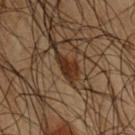Part of a total-body skin-imaging series; this lesion was reviewed on a skin check and was not flagged for biopsy. Approximately 2.5 mm at its widest. The total-body-photography lesion software estimated an outline eccentricity of about 0.8 (0 = round, 1 = elongated). The software also gave an average lesion color of about L≈21 a*≈15 b*≈23 (CIELAB), roughly 9 lightness units darker than nearby skin, and a lesion-to-skin contrast of about 10.5 (normalized; higher = more distinct). And it measured an automated nevus-likeness rating near 85 out of 100 and a detector confidence of about 95 out of 100 that the crop contains a lesion. A male patient, aged 48–52. The tile uses cross-polarized illumination. The lesion is on the arm. Cropped from a total-body skin-imaging series; the visible field is about 15 mm.